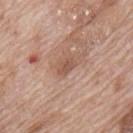<case>
  <biopsy_status>not biopsied; imaged during a skin examination</biopsy_status>
  <patient>
    <sex>male</sex>
    <age_approx>70</age_approx>
  </patient>
  <lesion_size>
    <long_diameter_mm_approx>2.5</long_diameter_mm_approx>
  </lesion_size>
  <lighting>white-light</lighting>
  <site>mid back</site>
  <automated_metrics>
    <area_mm2_approx>3.5</area_mm2_approx>
    <eccentricity>0.8</eccentricity>
    <shape_asymmetry>0.25</shape_asymmetry>
    <nevus_likeness_0_100>0</nevus_likeness_0_100>
    <lesion_detection_confidence_0_100>100</lesion_detection_confidence_0_100>
  </automated_metrics>
  <image>
    <source>total-body photography crop</source>
    <field_of_view_mm>15</field_of_view_mm>
  </image>
</case>